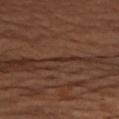biopsy status: catalogued during a skin exam; not biopsied
image source: 15 mm crop, total-body photography
patient: female, aged around 65
location: the left arm
tile lighting: cross-polarized illumination
lesion diameter: about 1 mm